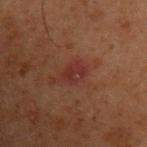This lesion was catalogued during total-body skin photography and was not selected for biopsy.
A 15 mm crop from a total-body photograph taken for skin-cancer surveillance.
From the arm.
A male subject roughly 60 years of age.